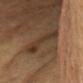This lesion was catalogued during total-body skin photography and was not selected for biopsy. On the head or neck. A 15 mm crop from a total-body photograph taken for skin-cancer surveillance. The tile uses cross-polarized illumination. A subject aged 58 to 62. Measured at roughly 5 mm in maximum diameter. An algorithmic analysis of the crop reported a mean CIELAB color near L≈35 a*≈15 b*≈27 and a lesion-to-skin contrast of about 7.5 (normalized; higher = more distinct). The software also gave border irregularity of about 4 on a 0–10 scale, a within-lesion color-variation index near 4.5/10, and peripheral color asymmetry of about 1.5. The software also gave a classifier nevus-likeness of about 0/100 and lesion-presence confidence of about 55/100.